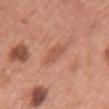- notes: imaged on a skin check; not biopsied
- location: the chest
- lesion size: ~3.5 mm (longest diameter)
- image source: total-body-photography crop, ~15 mm field of view
- tile lighting: white-light illumination
- automated metrics: an area of roughly 4 mm², an outline eccentricity of about 0.9 (0 = round, 1 = elongated), and two-axis asymmetry of about 0.3; a mean CIELAB color near L≈55 a*≈25 b*≈32, roughly 7 lightness units darker than nearby skin, and a lesion-to-skin contrast of about 5 (normalized; higher = more distinct); a border-irregularity rating of about 3/10 and radial color variation of about 0.5
- patient: female, about 60 years old